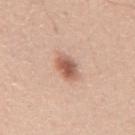A male subject aged 28 to 32. Imaged with white-light lighting. The lesion's longest dimension is about 3.5 mm. On the mid back. A roughly 15 mm field-of-view crop from a total-body skin photograph.